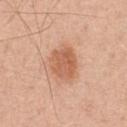notes: imaged on a skin check; not biopsied | body site: the chest | subject: male, approximately 50 years of age | illumination: white-light illumination | image source: total-body-photography crop, ~15 mm field of view | diameter: about 4 mm.